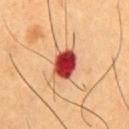This lesion was catalogued during total-body skin photography and was not selected for biopsy.
Longest diameter approximately 4 mm.
An algorithmic analysis of the crop reported a lesion color around L≈47 a*≈37 b*≈33 in CIELAB, roughly 22 lightness units darker than nearby skin, and a lesion-to-skin contrast of about 15 (normalized; higher = more distinct).
Imaged with cross-polarized lighting.
A 15 mm close-up extracted from a 3D total-body photography capture.
From the chest.
The patient is a male aged around 60.